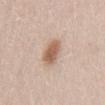The lesion was photographed on a routine skin check and not biopsied; there is no pathology result. A region of skin cropped from a whole-body photographic capture, roughly 15 mm wide. The lesion is located on the lower back. About 3 mm across. A female patient aged approximately 60.